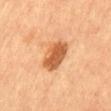This lesion was catalogued during total-body skin photography and was not selected for biopsy. About 4.5 mm across. The tile uses cross-polarized illumination. The patient is a male roughly 60 years of age. A region of skin cropped from a whole-body photographic capture, roughly 15 mm wide. The lesion is located on the abdomen.